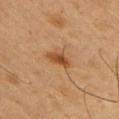follow-up: total-body-photography surveillance lesion; no biopsy
imaging modality: total-body-photography crop, ~15 mm field of view
anatomic site: the right upper arm
image-analysis metrics: an average lesion color of about L≈49 a*≈23 b*≈38 (CIELAB), roughly 11 lightness units darker than nearby skin, and a lesion-to-skin contrast of about 8.5 (normalized; higher = more distinct); a detector confidence of about 100 out of 100 that the crop contains a lesion
diameter: ≈3 mm
patient: male, aged 48–52